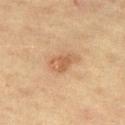Recorded during total-body skin imaging; not selected for excision or biopsy.
Measured at roughly 3.5 mm in maximum diameter.
A female patient, aged 63 to 67.
The lesion is located on the right thigh.
This is a cross-polarized tile.
A close-up tile cropped from a whole-body skin photograph, about 15 mm across.
The lesion-visualizer software estimated a lesion area of about 6 mm², an eccentricity of roughly 0.8, and two-axis asymmetry of about 0.3. The software also gave an automated nevus-likeness rating near 60 out of 100 and a lesion-detection confidence of about 100/100.